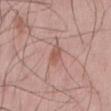| field | value |
|---|---|
| workup | imaged on a skin check; not biopsied |
| subject | male, aged 43–47 |
| image | total-body-photography crop, ~15 mm field of view |
| body site | the back |